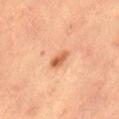Clinical impression: Captured during whole-body skin photography for melanoma surveillance; the lesion was not biopsied. Image and clinical context: The lesion is located on the lower back. About 2.5 mm across. This is a cross-polarized tile. Automated image analysis of the tile measured border irregularity of about 2.5 on a 0–10 scale, a color-variation rating of about 4.5/10, and radial color variation of about 2. And it measured a nevus-likeness score of about 85/100 and a detector confidence of about 100 out of 100 that the crop contains a lesion. A 15 mm close-up tile from a total-body photography series done for melanoma screening. The subject is a female aged around 60.